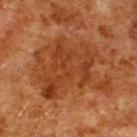| feature | finding |
|---|---|
| biopsy status | total-body-photography surveillance lesion; no biopsy |
| patient | male, aged 78–82 |
| image source | 15 mm crop, total-body photography |
| body site | the upper back |